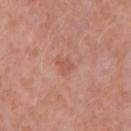workup: total-body-photography surveillance lesion; no biopsy | anatomic site: the right upper arm | imaging modality: ~15 mm crop, total-body skin-cancer survey | illumination: white-light illumination | subject: female, approximately 40 years of age | lesion size: ~2.5 mm (longest diameter) | automated lesion analysis: a footprint of about 3.5 mm² and an outline eccentricity of about 0.7 (0 = round, 1 = elongated); a lesion–skin lightness drop of about 7 and a lesion-to-skin contrast of about 5 (normalized; higher = more distinct); internal color variation of about 1 on a 0–10 scale and peripheral color asymmetry of about 0.5; a classifier nevus-likeness of about 0/100.